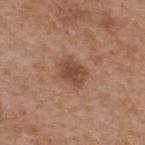<tbp_lesion>
  <biopsy_status>not biopsied; imaged during a skin examination</biopsy_status>
  <site>upper back</site>
  <image>
    <source>total-body photography crop</source>
    <field_of_view_mm>15</field_of_view_mm>
  </image>
  <patient>
    <sex>male</sex>
    <age_approx>55</age_approx>
  </patient>
</tbp_lesion>